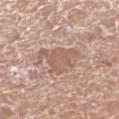Clinical impression: The lesion was photographed on a routine skin check and not biopsied; there is no pathology result.